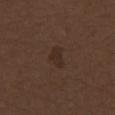Clinical impression:
Captured during whole-body skin photography for melanoma surveillance; the lesion was not biopsied.
Background:
This image is a 15 mm lesion crop taken from a total-body photograph. This is a white-light tile. The lesion is on the right thigh. A male subject approximately 70 years of age. The lesion-visualizer software estimated an area of roughly 3.5 mm² and a shape-asymmetry score of about 0.45 (0 = symmetric). And it measured an average lesion color of about L≈26 a*≈14 b*≈22 (CIELAB), about 5 CIELAB-L* units darker than the surrounding skin, and a lesion-to-skin contrast of about 6 (normalized; higher = more distinct). The lesion's longest dimension is about 2.5 mm.